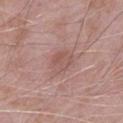follow-up: catalogued during a skin exam; not biopsied | body site: the leg | lighting: white-light illumination | size: ~3 mm (longest diameter) | subject: male, roughly 75 years of age | acquisition: 15 mm crop, total-body photography.